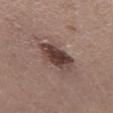No biopsy was performed on this lesion — it was imaged during a full skin examination and was not determined to be concerning.